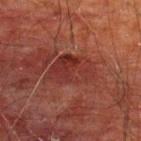| feature | finding |
|---|---|
| biopsy status | no biopsy performed (imaged during a skin exam) |
| illumination | cross-polarized illumination |
| patient | male, aged 58–62 |
| imaging modality | 15 mm crop, total-body photography |
| diameter | ≈5.5 mm |
| location | the upper back |